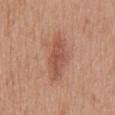Clinical impression: No biopsy was performed on this lesion — it was imaged during a full skin examination and was not determined to be concerning. Image and clinical context: A close-up tile cropped from a whole-body skin photograph, about 15 mm across. Approximately 7 mm at its widest. A female patient, in their 40s. On the mid back. Imaged with white-light lighting.